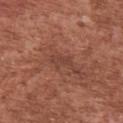The lesion was tiled from a total-body skin photograph and was not biopsied.
The patient is a male in their mid- to late 40s.
Approximately 2.5 mm at its widest.
Located on the chest.
The lesion-visualizer software estimated a lesion area of about 3 mm², an outline eccentricity of about 0.9 (0 = round, 1 = elongated), and a shape-asymmetry score of about 0.35 (0 = symmetric).
Captured under white-light illumination.
A region of skin cropped from a whole-body photographic capture, roughly 15 mm wide.